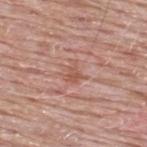Clinical impression:
Part of a total-body skin-imaging series; this lesion was reviewed on a skin check and was not flagged for biopsy.
Acquisition and patient details:
Captured under white-light illumination. A lesion tile, about 15 mm wide, cut from a 3D total-body photograph. Measured at roughly 2.5 mm in maximum diameter. On the upper back. The subject is a male roughly 65 years of age.